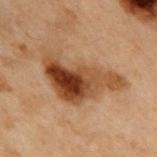Impression:
The lesion was photographed on a routine skin check and not biopsied; there is no pathology result.
Context:
A 15 mm crop from a total-body photograph taken for skin-cancer surveillance. Located on the upper back. A male patient approximately 55 years of age.